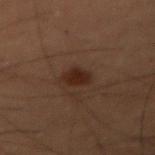Impression:
The lesion was photographed on a routine skin check and not biopsied; there is no pathology result.
Acquisition and patient details:
A lesion tile, about 15 mm wide, cut from a 3D total-body photograph. The lesion is on the right forearm. The lesion's longest dimension is about 2.5 mm. A male patient in their mid-50s. The tile uses cross-polarized illumination.